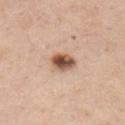follow-up: no biopsy performed (imaged during a skin exam); automated metrics: a footprint of about 6 mm², a shape eccentricity near 0.7, and two-axis asymmetry of about 0.2; patient: male, about 40 years old; size: ~3 mm (longest diameter); tile lighting: white-light; acquisition: ~15 mm tile from a whole-body skin photo; body site: the chest.